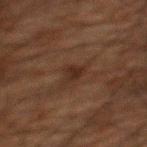<tbp_lesion>
<biopsy_status>not biopsied; imaged during a skin examination</biopsy_status>
<patient>
  <sex>male</sex>
  <age_approx>60</age_approx>
</patient>
<site>back</site>
<lesion_size>
  <long_diameter_mm_approx>2.5</long_diameter_mm_approx>
</lesion_size>
<lighting>cross-polarized</lighting>
<image>
  <source>total-body photography crop</source>
  <field_of_view_mm>15</field_of_view_mm>
</image>
</tbp_lesion>